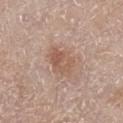Clinical impression: No biopsy was performed on this lesion — it was imaged during a full skin examination and was not determined to be concerning. Background: Located on the left upper arm. Cropped from a total-body skin-imaging series; the visible field is about 15 mm. Longest diameter approximately 4 mm. A male subject, aged approximately 80.